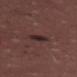{"biopsy_status": "not biopsied; imaged during a skin examination", "lighting": "white-light", "lesion_size": {"long_diameter_mm_approx": 2.0}, "patient": {"sex": "female", "age_approx": 50}, "automated_metrics": {"border_irregularity_0_10": 1.5, "color_variation_0_10": 5.0, "nevus_likeness_0_100": 55, "lesion_detection_confidence_0_100": 100}, "image": {"source": "total-body photography crop", "field_of_view_mm": 15}, "site": "right thigh"}